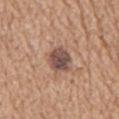{
  "biopsy_status": "not biopsied; imaged during a skin examination",
  "site": "chest",
  "patient": {
    "sex": "male",
    "age_approx": 65
  },
  "automated_metrics": {
    "area_mm2_approx": 7.5,
    "eccentricity": 0.75,
    "border_irregularity_0_10": 2.0,
    "color_variation_0_10": 5.0
  },
  "image": {
    "source": "total-body photography crop",
    "field_of_view_mm": 15
  },
  "lesion_size": {
    "long_diameter_mm_approx": 4.0
  },
  "lighting": "white-light"
}